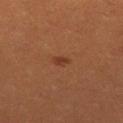No biopsy was performed on this lesion — it was imaged during a full skin examination and was not determined to be concerning. Captured under cross-polarized illumination. The patient is a female about 30 years old. From the left thigh. Cropped from a total-body skin-imaging series; the visible field is about 15 mm.